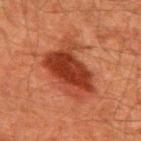follow-up = imaged on a skin check; not biopsied | subject = male, aged approximately 60 | lesion diameter = ≈7.5 mm | body site = the back | image source = 15 mm crop, total-body photography | illumination = cross-polarized illumination | TBP lesion metrics = a lesion area of about 26 mm² and a symmetry-axis asymmetry near 0.3; an average lesion color of about L≈32 a*≈26 b*≈29 (CIELAB), roughly 11 lightness units darker than nearby skin, and a normalized border contrast of about 9.5.